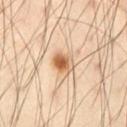The lesion was tiled from a total-body skin photograph and was not biopsied.
A close-up tile cropped from a whole-body skin photograph, about 15 mm across.
A male patient approximately 55 years of age.
Imaged with cross-polarized lighting.
An algorithmic analysis of the crop reported a lesion area of about 5.5 mm², an outline eccentricity of about 0.6 (0 = round, 1 = elongated), and a shape-asymmetry score of about 0.15 (0 = symmetric). It also reported an automated nevus-likeness rating near 95 out of 100 and a lesion-detection confidence of about 100/100.
Located on the right thigh.